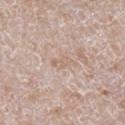Imaged during a routine full-body skin examination; the lesion was not biopsied and no histopathology is available. A male subject aged approximately 60. A close-up tile cropped from a whole-body skin photograph, about 15 mm across. On the right lower leg. Measured at roughly 3 mm in maximum diameter. The tile uses white-light illumination.